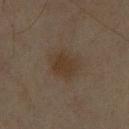{"biopsy_status": "not biopsied; imaged during a skin examination", "lesion_size": {"long_diameter_mm_approx": 3.5}, "lighting": "cross-polarized", "patient": {"sex": "male", "age_approx": 60}, "automated_metrics": {"area_mm2_approx": 9.5, "shape_asymmetry": 0.15, "vs_skin_contrast_norm": 7.0, "color_variation_0_10": 2.5, "peripheral_color_asymmetry": 1.0}, "site": "chest", "image": {"source": "total-body photography crop", "field_of_view_mm": 15}}